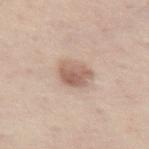The total-body-photography lesion software estimated a lesion color around L≈60 a*≈18 b*≈27 in CIELAB, roughly 12 lightness units darker than nearby skin, and a lesion-to-skin contrast of about 7.5 (normalized; higher = more distinct). The software also gave a border-irregularity index near 1.5/10, a color-variation rating of about 4.5/10, and radial color variation of about 1.5. And it measured a nevus-likeness score of about 40/100 and lesion-presence confidence of about 100/100. A male patient, in their mid- to late 70s. Measured at roughly 3 mm in maximum diameter. A 15 mm close-up tile from a total-body photography series done for melanoma screening. Captured under white-light illumination. Located on the leg.